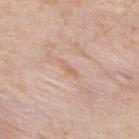A male patient, roughly 35 years of age.
On the upper back.
The lesion-visualizer software estimated a lesion color around L≈64 a*≈19 b*≈31 in CIELAB and a lesion–skin lightness drop of about 6. The software also gave a border-irregularity rating of about 4.5/10 and radial color variation of about 0. The analysis additionally found a classifier nevus-likeness of about 0/100 and lesion-presence confidence of about 95/100.
The recorded lesion diameter is about 3 mm.
A region of skin cropped from a whole-body photographic capture, roughly 15 mm wide.